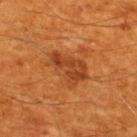notes: total-body-photography surveillance lesion; no biopsy
automated lesion analysis: a lesion area of about 12 mm², a shape eccentricity near 0.8, and a symmetry-axis asymmetry near 0.3; about 8 CIELAB-L* units darker than the surrounding skin and a lesion-to-skin contrast of about 7 (normalized; higher = more distinct); a border-irregularity rating of about 3.5/10
lesion size: ≈5 mm
anatomic site: the upper back
subject: male, aged 58–62
acquisition: ~15 mm tile from a whole-body skin photo